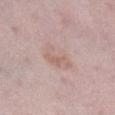The lesion was photographed on a routine skin check and not biopsied; there is no pathology result.
An algorithmic analysis of the crop reported a mean CIELAB color near L≈63 a*≈18 b*≈23, a lesion–skin lightness drop of about 6, and a normalized border contrast of about 5. The analysis additionally found an automated nevus-likeness rating near 20 out of 100 and a detector confidence of about 100 out of 100 that the crop contains a lesion.
Located on the right lower leg.
Cropped from a whole-body photographic skin survey; the tile spans about 15 mm.
A female patient, in their mid-40s.
Imaged with white-light lighting.
The lesion's longest dimension is about 4 mm.